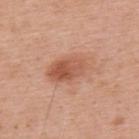subject = male, about 60 years old; anatomic site = the upper back; illumination = white-light illumination; image source = 15 mm crop, total-body photography; diameter = about 5.5 mm.